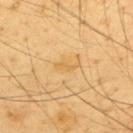Q: Was this lesion biopsied?
A: catalogued during a skin exam; not biopsied
Q: Illumination type?
A: cross-polarized illumination
Q: What did automated image analysis measure?
A: an outline eccentricity of about 0.95 (0 = round, 1 = elongated) and a shape-asymmetry score of about 0.4 (0 = symmetric); a lesion color around L≈69 a*≈18 b*≈48 in CIELAB, about 6 CIELAB-L* units darker than the surrounding skin, and a normalized border contrast of about 5; border irregularity of about 4.5 on a 0–10 scale and a color-variation rating of about 0/10; an automated nevus-likeness rating near 0 out of 100 and a detector confidence of about 100 out of 100 that the crop contains a lesion
Q: Lesion location?
A: the upper back
Q: How large is the lesion?
A: ~3 mm (longest diameter)
Q: Patient demographics?
A: male, about 65 years old
Q: How was this image acquired?
A: total-body-photography crop, ~15 mm field of view